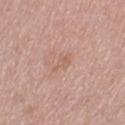Recorded during total-body skin imaging; not selected for excision or biopsy. A female subject, aged 28–32. Cropped from a whole-body photographic skin survey; the tile spans about 15 mm. Measured at roughly 2.5 mm in maximum diameter. This is a white-light tile. Automated image analysis of the tile measured peripheral color asymmetry of about 0.5. The lesion is on the left thigh.